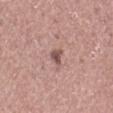Impression:
The lesion was photographed on a routine skin check and not biopsied; there is no pathology result.
Context:
A 15 mm close-up tile from a total-body photography series done for melanoma screening. The lesion is located on the left thigh. Automated image analysis of the tile measured a lesion area of about 3 mm². The software also gave border irregularity of about 3.5 on a 0–10 scale, a color-variation rating of about 1.5/10, and peripheral color asymmetry of about 0.5. It also reported lesion-presence confidence of about 100/100. A female subject aged 48 to 52. Approximately 2.5 mm at its widest. This is a white-light tile.